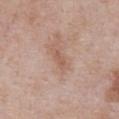notes — catalogued during a skin exam; not biopsied
site — the abdomen
lesion size — ≈3 mm
subject — male, in their mid- to late 50s
acquisition — ~15 mm tile from a whole-body skin photo
illumination — white-light illumination